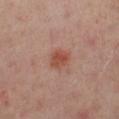Q: Was this lesion biopsied?
A: total-body-photography surveillance lesion; no biopsy
Q: What is the anatomic site?
A: the leg
Q: What kind of image is this?
A: ~15 mm crop, total-body skin-cancer survey
Q: What are the patient's age and sex?
A: female, aged 38 to 42